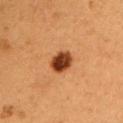Impression:
The lesion was tiled from a total-body skin photograph and was not biopsied.